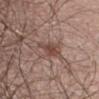The lesion was tiled from a total-body skin photograph and was not biopsied. An algorithmic analysis of the crop reported a footprint of about 5 mm². And it measured a classifier nevus-likeness of about 80/100 and a lesion-detection confidence of about 100/100. The lesion is on the chest. A male patient approximately 50 years of age. This image is a 15 mm lesion crop taken from a total-body photograph.